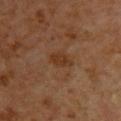A male subject, aged 58 to 62.
Approximately 3 mm at its widest.
The tile uses cross-polarized illumination.
A 15 mm crop from a total-body photograph taken for skin-cancer surveillance.
From the upper back.